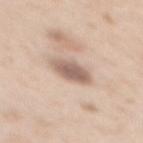workup: total-body-photography surveillance lesion; no biopsy | automated lesion analysis: a lesion area of about 7.5 mm² and a shape eccentricity near 0.8; a lesion color around L≈61 a*≈16 b*≈25 in CIELAB, roughly 14 lightness units darker than nearby skin, and a lesion-to-skin contrast of about 8.5 (normalized; higher = more distinct) | image source: total-body-photography crop, ~15 mm field of view | size: about 4 mm | location: the mid back | subject: female, aged approximately 50.